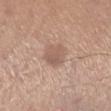Case summary:
• biopsy status · catalogued during a skin exam; not biopsied
• subject · male, approximately 40 years of age
• body site · the right lower leg
• size · ~3.5 mm (longest diameter)
• lighting · white-light
• imaging modality · 15 mm crop, total-body photography